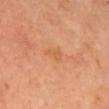Q: Was this lesion biopsied?
A: imaged on a skin check; not biopsied
Q: Who is the patient?
A: male, about 70 years old
Q: What is the lesion's diameter?
A: ≈3 mm
Q: Automated lesion metrics?
A: a shape eccentricity near 0.85 and a symmetry-axis asymmetry near 0.35; a border-irregularity rating of about 3.5/10 and a color-variation rating of about 1/10; an automated nevus-likeness rating near 0 out of 100 and a lesion-detection confidence of about 100/100
Q: Where on the body is the lesion?
A: the head or neck
Q: How was the tile lit?
A: cross-polarized illumination
Q: How was this image acquired?
A: 15 mm crop, total-body photography